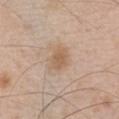{
  "biopsy_status": "not biopsied; imaged during a skin examination",
  "patient": {
    "sex": "male",
    "age_approx": 50
  },
  "image": {
    "source": "total-body photography crop",
    "field_of_view_mm": 15
  },
  "site": "front of the torso",
  "lesion_size": {
    "long_diameter_mm_approx": 3.0
  },
  "lighting": "white-light"
}